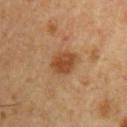The subject is a male aged around 55. The total-body-photography lesion software estimated a footprint of about 8.5 mm², an outline eccentricity of about 0.6 (0 = round, 1 = elongated), and a symmetry-axis asymmetry near 0.2. It also reported a mean CIELAB color near L≈47 a*≈22 b*≈35, about 10 CIELAB-L* units darker than the surrounding skin, and a normalized lesion–skin contrast near 8. It also reported an automated nevus-likeness rating near 85 out of 100. A 15 mm close-up tile from a total-body photography series done for melanoma screening. The lesion is located on the right upper arm. The recorded lesion diameter is about 3.5 mm. This is a cross-polarized tile.